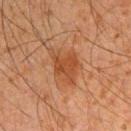<lesion>
  <biopsy_status>not biopsied; imaged during a skin examination</biopsy_status>
  <automated_metrics>
    <area_mm2_approx>11.0</area_mm2_approx>
    <shape_asymmetry>0.35</shape_asymmetry>
    <cielab_L>37</cielab_L>
    <cielab_a>21</cielab_a>
    <cielab_b>30</cielab_b>
    <vs_skin_darker_L>7.0</vs_skin_darker_L>
    <vs_skin_contrast_norm>7.0</vs_skin_contrast_norm>
    <border_irregularity_0_10>4.5</border_irregularity_0_10>
    <peripheral_color_asymmetry>1.0</peripheral_color_asymmetry>
  </automated_metrics>
  <lighting>cross-polarized</lighting>
  <site>right upper arm</site>
  <image>
    <source>total-body photography crop</source>
    <field_of_view_mm>15</field_of_view_mm>
  </image>
  <patient>
    <sex>male</sex>
    <age_approx>35</age_approx>
  </patient>
  <lesion_size>
    <long_diameter_mm_approx>5.0</long_diameter_mm_approx>
  </lesion_size>
</lesion>